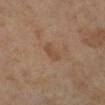This lesion was catalogued during total-body skin photography and was not selected for biopsy. A close-up tile cropped from a whole-body skin photograph, about 15 mm across. A female patient, in their 70s. From the left lower leg.